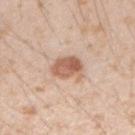Imaged during a routine full-body skin examination; the lesion was not biopsied and no histopathology is available.
The patient is a male roughly 25 years of age.
On the right forearm.
A 15 mm close-up tile from a total-body photography series done for melanoma screening.